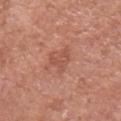Q: Was a biopsy performed?
A: imaged on a skin check; not biopsied
Q: What did automated image analysis measure?
A: a lesion color around L≈53 a*≈25 b*≈30 in CIELAB, a lesion–skin lightness drop of about 7, and a normalized lesion–skin contrast near 5; a border-irregularity index near 5.5/10, a color-variation rating of about 1.5/10, and radial color variation of about 0.5; a lesion-detection confidence of about 100/100
Q: What lighting was used for the tile?
A: white-light
Q: What is the anatomic site?
A: the arm
Q: Who is the patient?
A: male, aged around 75
Q: Lesion size?
A: ≈3 mm
Q: What kind of image is this?
A: 15 mm crop, total-body photography